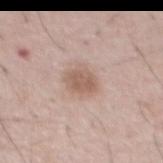Part of a total-body skin-imaging series; this lesion was reviewed on a skin check and was not flagged for biopsy. Automated image analysis of the tile measured a footprint of about 6 mm², an eccentricity of roughly 0.65, and a symmetry-axis asymmetry near 0.2. The software also gave roughly 10 lightness units darker than nearby skin and a normalized lesion–skin contrast near 7. The analysis additionally found a nevus-likeness score of about 55/100 and a detector confidence of about 100 out of 100 that the crop contains a lesion. Captured under white-light illumination. A 15 mm close-up tile from a total-body photography series done for melanoma screening. Longest diameter approximately 3 mm. The subject is a male aged approximately 55.